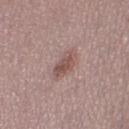{
  "lighting": "white-light",
  "image": {
    "source": "total-body photography crop",
    "field_of_view_mm": 15
  },
  "patient": {
    "sex": "female",
    "age_approx": 50
  },
  "site": "right thigh",
  "automated_metrics": {
    "area_mm2_approx": 5.5,
    "eccentricity": 0.8,
    "shape_asymmetry": 0.15,
    "vs_skin_darker_L": 10.0,
    "vs_skin_contrast_norm": 7.0,
    "border_irregularity_0_10": 2.0,
    "color_variation_0_10": 3.0,
    "peripheral_color_asymmetry": 1.0
  }
}